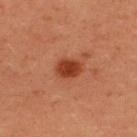Image and clinical context: The tile uses cross-polarized illumination. A 15 mm close-up extracted from a 3D total-body photography capture. The recorded lesion diameter is about 3 mm. A female patient, in their mid-30s. The lesion is located on the upper back.